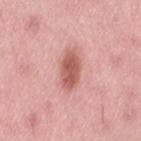notes: catalogued during a skin exam; not biopsied | patient: female, roughly 50 years of age | image: 15 mm crop, total-body photography | lesion diameter: ≈4.5 mm | anatomic site: the lower back | illumination: white-light illumination | TBP lesion metrics: a mean CIELAB color near L≈58 a*≈28 b*≈26, about 14 CIELAB-L* units darker than the surrounding skin, and a normalized lesion–skin contrast near 9; a border-irregularity index near 2/10, a within-lesion color-variation index near 3/10, and peripheral color asymmetry of about 1; an automated nevus-likeness rating near 90 out of 100.